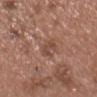Imaged during a routine full-body skin examination; the lesion was not biopsied and no histopathology is available. The lesion is located on the chest. A 15 mm close-up tile from a total-body photography series done for melanoma screening. A male subject approximately 55 years of age. Measured at roughly 2.5 mm in maximum diameter. The tile uses white-light illumination.